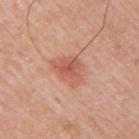Recorded during total-body skin imaging; not selected for excision or biopsy. The patient is a male aged 48–52. Longest diameter approximately 4.5 mm. The lesion is on the right upper arm. A roughly 15 mm field-of-view crop from a total-body skin photograph.